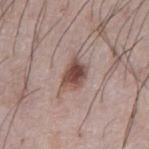Assessment: Captured during whole-body skin photography for melanoma surveillance; the lesion was not biopsied. Clinical summary: The subject is a male in their mid- to late 70s. Automated image analysis of the tile measured an area of roughly 7 mm², an outline eccentricity of about 0.7 (0 = round, 1 = elongated), and a symmetry-axis asymmetry near 0.3. The software also gave a mean CIELAB color near L≈48 a*≈18 b*≈23, roughly 14 lightness units darker than nearby skin, and a lesion-to-skin contrast of about 10 (normalized; higher = more distinct). It also reported lesion-presence confidence of about 100/100. About 3.5 mm across. Captured under white-light illumination. Located on the abdomen. A region of skin cropped from a whole-body photographic capture, roughly 15 mm wide.